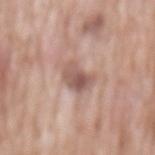A male patient, aged 58 to 62. The tile uses white-light illumination. This image is a 15 mm lesion crop taken from a total-body photograph. The lesion is located on the front of the torso.